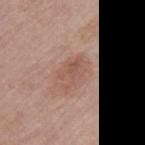Q: Was a biopsy performed?
A: total-body-photography surveillance lesion; no biopsy
Q: What are the patient's age and sex?
A: female, roughly 50 years of age
Q: What is the anatomic site?
A: the left upper arm
Q: What is the imaging modality?
A: ~15 mm crop, total-body skin-cancer survey